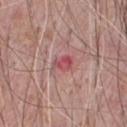Q: What did automated image analysis measure?
A: border irregularity of about 2.5 on a 0–10 scale, a color-variation rating of about 4.5/10, and peripheral color asymmetry of about 1.5; a classifier nevus-likeness of about 0/100 and a detector confidence of about 100 out of 100 that the crop contains a lesion
Q: Lesion size?
A: ~2.5 mm (longest diameter)
Q: How was the tile lit?
A: white-light
Q: Where on the body is the lesion?
A: the chest
Q: What is the imaging modality?
A: ~15 mm tile from a whole-body skin photo
Q: Patient demographics?
A: male, in their 70s